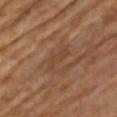Automated tile analysis of the lesion measured a lesion area of about 3.5 mm², an eccentricity of roughly 0.95, and a shape-asymmetry score of about 0.55 (0 = symmetric). And it measured an average lesion color of about L≈41 a*≈20 b*≈30 (CIELAB) and roughly 5 lightness units darker than nearby skin. It also reported a border-irregularity rating of about 6.5/10 and radial color variation of about 0. Located on the chest. This image is a 15 mm lesion crop taken from a total-body photograph. A female subject, aged 63 to 67. This is a cross-polarized tile.